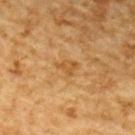Clinical impression: Imaged during a routine full-body skin examination; the lesion was not biopsied and no histopathology is available. Acquisition and patient details: A male patient roughly 85 years of age or older. The lesion is on the upper back. A 15 mm close-up tile from a total-body photography series done for melanoma screening. This is a cross-polarized tile. The recorded lesion diameter is about 3 mm. The total-body-photography lesion software estimated an area of roughly 3.5 mm², an eccentricity of roughly 0.75, and a shape-asymmetry score of about 0.5 (0 = symmetric). The analysis additionally found an average lesion color of about L≈47 a*≈19 b*≈39 (CIELAB), about 7 CIELAB-L* units darker than the surrounding skin, and a lesion-to-skin contrast of about 6 (normalized; higher = more distinct). And it measured an automated nevus-likeness rating near 0 out of 100.